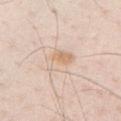biopsy status: imaged on a skin check; not biopsied.